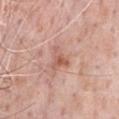Clinical impression:
No biopsy was performed on this lesion — it was imaged during a full skin examination and was not determined to be concerning.
Background:
A close-up tile cropped from a whole-body skin photograph, about 15 mm across. A male subject in their 50s. Imaged with white-light lighting. From the chest.